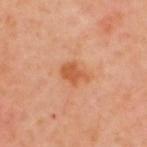biopsy status: catalogued during a skin exam; not biopsied | patient: male, in their mid-60s | size: ~3.5 mm (longest diameter) | location: the upper back | acquisition: ~15 mm crop, total-body skin-cancer survey | tile lighting: cross-polarized illumination | automated metrics: a border-irregularity rating of about 3/10, internal color variation of about 2 on a 0–10 scale, and radial color variation of about 0.5; a lesion-detection confidence of about 100/100.